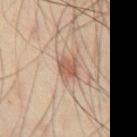Q: Is there a histopathology result?
A: no biopsy performed (imaged during a skin exam)
Q: Lesion size?
A: ≈3 mm
Q: Where on the body is the lesion?
A: the abdomen
Q: How was this image acquired?
A: ~15 mm crop, total-body skin-cancer survey
Q: What are the patient's age and sex?
A: male, roughly 60 years of age
Q: Illumination type?
A: cross-polarized
Q: What did automated image analysis measure?
A: an average lesion color of about L≈50 a*≈18 b*≈26 (CIELAB) and a normalized lesion–skin contrast near 7.5; a detector confidence of about 100 out of 100 that the crop contains a lesion